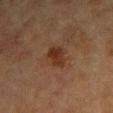<lesion>
<biopsy_status>not biopsied; imaged during a skin examination</biopsy_status>
<lesion_size>
  <long_diameter_mm_approx>3.5</long_diameter_mm_approx>
</lesion_size>
<lighting>cross-polarized</lighting>
<patient>
  <sex>female</sex>
  <age_approx>80</age_approx>
</patient>
<image>
  <source>total-body photography crop</source>
  <field_of_view_mm>15</field_of_view_mm>
</image>
<site>left forearm</site>
</lesion>